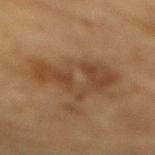workup: no biopsy performed (imaged during a skin exam) | acquisition: 15 mm crop, total-body photography | automated lesion analysis: a footprint of about 21 mm² and two-axis asymmetry of about 0.35; an average lesion color of about L≈37 a*≈16 b*≈30 (CIELAB), about 7 CIELAB-L* units darker than the surrounding skin, and a normalized border contrast of about 6.5; a classifier nevus-likeness of about 0/100 and a detector confidence of about 100 out of 100 that the crop contains a lesion | subject: male, approximately 85 years of age or older | site: the mid back | size: ≈7.5 mm | lighting: cross-polarized illumination.